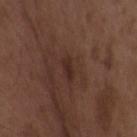| field | value |
|---|---|
| lighting | white-light |
| TBP lesion metrics | an area of roughly 3.5 mm², an outline eccentricity of about 0.9 (0 = round, 1 = elongated), and a shape-asymmetry score of about 0.2 (0 = symmetric) |
| patient | female, about 35 years old |
| site | the upper back |
| image source | ~15 mm crop, total-body skin-cancer survey |